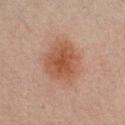workup — total-body-photography surveillance lesion; no biopsy | location — the chest | subject — male, aged around 30 | size — ~6 mm (longest diameter) | acquisition — 15 mm crop, total-body photography | tile lighting — cross-polarized illumination | automated metrics — a shape eccentricity near 0.6; a lesion color around L≈43 a*≈19 b*≈27 in CIELAB, about 8 CIELAB-L* units darker than the surrounding skin, and a normalized border contrast of about 7.5; lesion-presence confidence of about 100/100.